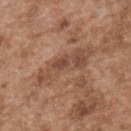biopsy_status: not biopsied; imaged during a skin examination
site: upper back
lighting: white-light
automated_metrics:
  area_mm2_approx: 13.0
  eccentricity: 0.95
  shape_asymmetry: 0.35
  cielab_L: 48
  cielab_a: 21
  cielab_b: 29
  vs_skin_darker_L: 8.0
  vs_skin_contrast_norm: 6.0
  color_variation_0_10: 5.0
  nevus_likeness_0_100: 0
  lesion_detection_confidence_0_100: 65
patient:
  sex: male
  age_approx: 65
image:
  source: total-body photography crop
  field_of_view_mm: 15